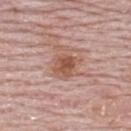<lesion>
<biopsy_status>not biopsied; imaged during a skin examination</biopsy_status>
<lesion_size>
  <long_diameter_mm_approx>3.5</long_diameter_mm_approx>
</lesion_size>
<patient>
  <sex>female</sex>
  <age_approx>65</age_approx>
</patient>
<image>
  <source>total-body photography crop</source>
  <field_of_view_mm>15</field_of_view_mm>
</image>
<site>upper back</site>
</lesion>